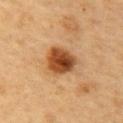The lesion is on the upper back.
Imaged with cross-polarized lighting.
Cropped from a whole-body photographic skin survey; the tile spans about 15 mm.
A female patient aged 58–62.
Measured at roughly 4 mm in maximum diameter.